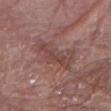<record>
<biopsy_status>not biopsied; imaged during a skin examination</biopsy_status>
<site>left arm</site>
<lesion_size>
  <long_diameter_mm_approx>5.0</long_diameter_mm_approx>
</lesion_size>
<automated_metrics>
  <cielab_L>45</cielab_L>
  <cielab_a>21</cielab_a>
  <cielab_b>22</cielab_b>
  <vs_skin_darker_L>7.0</vs_skin_darker_L>
  <vs_skin_contrast_norm>6.0</vs_skin_contrast_norm>
  <border_irregularity_0_10>6.5</border_irregularity_0_10>
  <color_variation_0_10>3.0</color_variation_0_10>
  <peripheral_color_asymmetry>1.0</peripheral_color_asymmetry>
</automated_metrics>
<lighting>white-light</lighting>
<patient>
  <sex>male</sex>
  <age_approx>80</age_approx>
</patient>
<image>
  <source>total-body photography crop</source>
  <field_of_view_mm>15</field_of_view_mm>
</image>
</record>